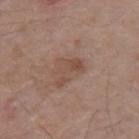This lesion was catalogued during total-body skin photography and was not selected for biopsy. Cropped from a total-body skin-imaging series; the visible field is about 15 mm. From the upper back. A male subject roughly 70 years of age. Automated tile analysis of the lesion measured an average lesion color of about L≈48 a*≈18 b*≈26 (CIELAB), about 7 CIELAB-L* units darker than the surrounding skin, and a normalized border contrast of about 6. The analysis additionally found border irregularity of about 8.5 on a 0–10 scale, a within-lesion color-variation index near 1.5/10, and a peripheral color-asymmetry measure near 0.5. Captured under white-light illumination.